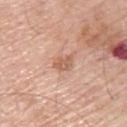Part of a total-body skin-imaging series; this lesion was reviewed on a skin check and was not flagged for biopsy. On the upper back. The patient is a male aged approximately 60. A 15 mm close-up tile from a total-body photography series done for melanoma screening.